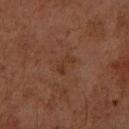Q: Was this lesion biopsied?
A: no biopsy performed (imaged during a skin exam)
Q: Patient demographics?
A: female, roughly 60 years of age
Q: What is the imaging modality?
A: ~15 mm crop, total-body skin-cancer survey
Q: Where on the body is the lesion?
A: the right upper arm